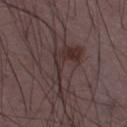Impression:
Imaged during a routine full-body skin examination; the lesion was not biopsied and no histopathology is available.
Acquisition and patient details:
Automated image analysis of the tile measured a lesion area of about 16 mm² and an outline eccentricity of about 0.9 (0 = round, 1 = elongated). Cropped from a total-body skin-imaging series; the visible field is about 15 mm. From the right thigh. This is a white-light tile. A male patient, aged 48 to 52.